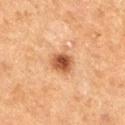The lesion was tiled from a total-body skin photograph and was not biopsied.
A 15 mm close-up tile from a total-body photography series done for melanoma screening.
The recorded lesion diameter is about 3 mm.
A male subject, in their mid-70s.
The lesion-visualizer software estimated a lesion area of about 6 mm², an outline eccentricity of about 0.25 (0 = round, 1 = elongated), and two-axis asymmetry of about 0.2. The software also gave a lesion–skin lightness drop of about 13 and a lesion-to-skin contrast of about 9.5 (normalized; higher = more distinct). The analysis additionally found border irregularity of about 2 on a 0–10 scale, a within-lesion color-variation index near 5/10, and peripheral color asymmetry of about 2. The analysis additionally found a nevus-likeness score of about 95/100 and lesion-presence confidence of about 100/100.
On the right thigh.